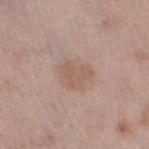Recorded during total-body skin imaging; not selected for excision or biopsy. On the right thigh. The recorded lesion diameter is about 3.5 mm. The total-body-photography lesion software estimated an average lesion color of about L≈58 a*≈17 b*≈25 (CIELAB) and a normalized border contrast of about 5. The software also gave border irregularity of about 2 on a 0–10 scale, internal color variation of about 2.5 on a 0–10 scale, and a peripheral color-asymmetry measure near 1. And it measured a nevus-likeness score of about 5/100 and a lesion-detection confidence of about 100/100. This image is a 15 mm lesion crop taken from a total-body photograph. This is a white-light tile. A female patient approximately 50 years of age.